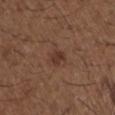biopsy_status: not biopsied; imaged during a skin examination
patient:
  sex: male
  age_approx: 50
lighting: white-light
automated_metrics:
  area_mm2_approx: 4.0
  eccentricity: 0.65
  shape_asymmetry: 0.3
  cielab_L: 35
  cielab_a: 19
  cielab_b: 24
  vs_skin_darker_L: 7.0
  vs_skin_contrast_norm: 7.0
  border_irregularity_0_10: 2.5
  color_variation_0_10: 3.0
  peripheral_color_asymmetry: 1.0
  nevus_likeness_0_100: 35
  lesion_detection_confidence_0_100: 100
site: right upper arm
image:
  source: total-body photography crop
  field_of_view_mm: 15